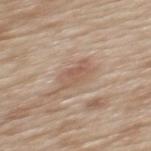Recorded during total-body skin imaging; not selected for excision or biopsy.
A female patient aged 38–42.
On the upper back.
The total-body-photography lesion software estimated an average lesion color of about L≈57 a*≈17 b*≈28 (CIELAB) and about 8 CIELAB-L* units darker than the surrounding skin. It also reported a border-irregularity rating of about 3/10, a within-lesion color-variation index near 3.5/10, and peripheral color asymmetry of about 1.
Captured under white-light illumination.
This image is a 15 mm lesion crop taken from a total-body photograph.